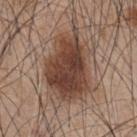Part of a total-body skin-imaging series; this lesion was reviewed on a skin check and was not flagged for biopsy.
A 15 mm close-up tile from a total-body photography series done for melanoma screening.
Measured at roughly 10 mm in maximum diameter.
Captured under white-light illumination.
A male subject roughly 45 years of age.
On the mid back.